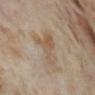The lesion was photographed on a routine skin check and not biopsied; there is no pathology result. Captured under cross-polarized illumination. Measured at roughly 4.5 mm in maximum diameter. The patient is a female aged 53–57. Automated image analysis of the tile measured a border-irregularity rating of about 7.5/10 and radial color variation of about 1.5. A 15 mm close-up extracted from a 3D total-body photography capture. From the leg.